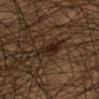Assessment: Recorded during total-body skin imaging; not selected for excision or biopsy. Image and clinical context: The tile uses cross-polarized illumination. A 15 mm close-up tile from a total-body photography series done for melanoma screening. The lesion's longest dimension is about 4 mm. The lesion is located on the left lower leg. The total-body-photography lesion software estimated a lesion color around L≈21 a*≈15 b*≈22 in CIELAB and roughly 8 lightness units darker than nearby skin. The software also gave a nevus-likeness score of about 100/100 and lesion-presence confidence of about 95/100. A male patient, approximately 45 years of age.